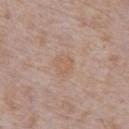Clinical impression: Imaged during a routine full-body skin examination; the lesion was not biopsied and no histopathology is available. Context: A 15 mm close-up extracted from a 3D total-body photography capture. Captured under white-light illumination. A male subject aged 68 to 72. Approximately 2.5 mm at its widest. The lesion is on the chest. The lesion-visualizer software estimated a nevus-likeness score of about 0/100 and a lesion-detection confidence of about 100/100.